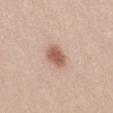- workup: total-body-photography surveillance lesion; no biopsy
- patient: female, roughly 25 years of age
- image-analysis metrics: lesion-presence confidence of about 100/100
- acquisition: 15 mm crop, total-body photography
- location: the right thigh